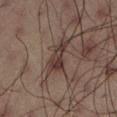Recorded during total-body skin imaging; not selected for excision or biopsy.
Automated tile analysis of the lesion measured an area of roughly 4.5 mm² and an eccentricity of roughly 0.9. The software also gave a detector confidence of about 100 out of 100 that the crop contains a lesion.
A male subject, approximately 60 years of age.
A 15 mm close-up tile from a total-body photography series done for melanoma screening.
About 3 mm across.